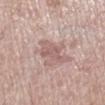Recorded during total-body skin imaging; not selected for excision or biopsy.
This image is a 15 mm lesion crop taken from a total-body photograph.
The subject is a female aged around 70.
Located on the right lower leg.
The tile uses white-light illumination.
Approximately 5 mm at its widest.